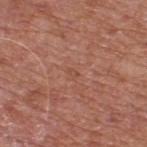The lesion was photographed on a routine skin check and not biopsied; there is no pathology result.
Cropped from a whole-body photographic skin survey; the tile spans about 15 mm.
A male patient in their mid- to late 60s.
The lesion is on the back.